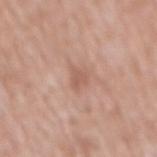This lesion was catalogued during total-body skin photography and was not selected for biopsy. The lesion is on the mid back. A lesion tile, about 15 mm wide, cut from a 3D total-body photograph. A male patient, aged 53–57.